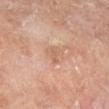Recorded during total-body skin imaging; not selected for excision or biopsy. The lesion is on the left lower leg. The lesion-visualizer software estimated an eccentricity of roughly 0.8 and a symmetry-axis asymmetry near 0.45. The analysis additionally found a normalized lesion–skin contrast near 4.5. The tile uses cross-polarized illumination. Cropped from a whole-body photographic skin survey; the tile spans about 15 mm. The lesion's longest dimension is about 2.5 mm. A male subject about 65 years old.